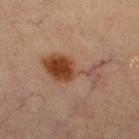Part of a total-body skin-imaging series; this lesion was reviewed on a skin check and was not flagged for biopsy. A female patient aged 63–67. The lesion is on the right lower leg. A roughly 15 mm field-of-view crop from a total-body skin photograph. The recorded lesion diameter is about 7.5 mm.